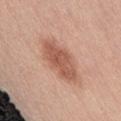Assessment: Part of a total-body skin-imaging series; this lesion was reviewed on a skin check and was not flagged for biopsy. Background: A 15 mm close-up tile from a total-body photography series done for melanoma screening. The lesion is on the abdomen. The subject is a female aged approximately 40.